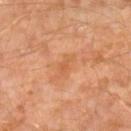Assessment: Part of a total-body skin-imaging series; this lesion was reviewed on a skin check and was not flagged for biopsy. Background: Captured under cross-polarized illumination. A male subject approximately 30 years of age. A lesion tile, about 15 mm wide, cut from a 3D total-body photograph. Approximately 3 mm at its widest. The lesion is on the left leg.